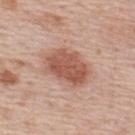biopsy status — catalogued during a skin exam; not biopsied
automated metrics — a border-irregularity index near 2/10 and a peripheral color-asymmetry measure near 1
acquisition — 15 mm crop, total-body photography
location — the upper back
size — ~6 mm (longest diameter)
subject — male, aged 73–77
tile lighting — white-light illumination